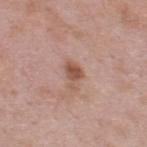{"biopsy_status": "not biopsied; imaged during a skin examination", "patient": {"sex": "male", "age_approx": 55}, "site": "upper back", "image": {"source": "total-body photography crop", "field_of_view_mm": 15}}